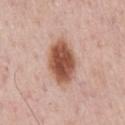workup = imaged on a skin check; not biopsied | lesion diameter = ~6 mm (longest diameter) | tile lighting = white-light | patient = male, in their 60s | acquisition = 15 mm crop, total-body photography | anatomic site = the chest.